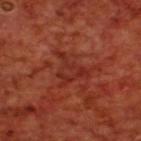Q: Was a biopsy performed?
A: imaged on a skin check; not biopsied
Q: What is the anatomic site?
A: the upper back
Q: What is the imaging modality?
A: total-body-photography crop, ~15 mm field of view
Q: What are the patient's age and sex?
A: male, aged approximately 70
Q: Lesion size?
A: ≈4.5 mm
Q: What lighting was used for the tile?
A: cross-polarized